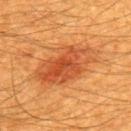  biopsy_status: not biopsied; imaged during a skin examination
  patient:
    sex: male
    age_approx: 60
  lesion_size:
    long_diameter_mm_approx: 7.5
  site: back
  image:
    source: total-body photography crop
    field_of_view_mm: 15